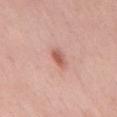This lesion was catalogued during total-body skin photography and was not selected for biopsy.
The subject is a male aged 53–57.
A 15 mm crop from a total-body photograph taken for skin-cancer surveillance.
Located on the chest.
The lesion-visualizer software estimated a footprint of about 3.5 mm², an outline eccentricity of about 0.8 (0 = round, 1 = elongated), and a shape-asymmetry score of about 0.3 (0 = symmetric).
The tile uses white-light illumination.
Longest diameter approximately 2.5 mm.